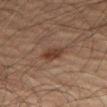• follow-up — catalogued during a skin exam; not biopsied
• size — ≈3 mm
• subject — male, in their 70s
• site — the leg
• imaging modality — ~15 mm tile from a whole-body skin photo
• lighting — cross-polarized illumination
• image-analysis metrics — a shape eccentricity near 0.8 and two-axis asymmetry of about 0.3